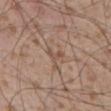No biopsy was performed on this lesion — it was imaged during a full skin examination and was not determined to be concerning.
Approximately 2.5 mm at its widest.
A male patient roughly 55 years of age.
A roughly 15 mm field-of-view crop from a total-body skin photograph.
This is a white-light tile.
From the front of the torso.